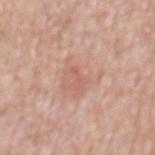biopsy_status: not biopsied; imaged during a skin examination
lesion_size:
  long_diameter_mm_approx: 2.5
site: back
image:
  source: total-body photography crop
  field_of_view_mm: 15
lighting: white-light
patient:
  sex: male
  age_approx: 55